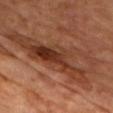workup=no biopsy performed (imaged during a skin exam)
illumination=cross-polarized
lesion diameter=about 11.5 mm
imaging modality=total-body-photography crop, ~15 mm field of view
subject=male, about 65 years old